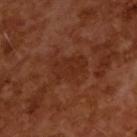Imaged during a routine full-body skin examination; the lesion was not biopsied and no histopathology is available. Automated image analysis of the tile measured a footprint of about 10 mm² and an outline eccentricity of about 0.7 (0 = round, 1 = elongated). Imaged with cross-polarized lighting. Longest diameter approximately 4 mm. This image is a 15 mm lesion crop taken from a total-body photograph. A male subject, aged 63–67.